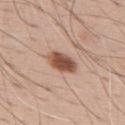Captured during whole-body skin photography for melanoma surveillance; the lesion was not biopsied.
Cropped from a total-body skin-imaging series; the visible field is about 15 mm.
The lesion's longest dimension is about 4 mm.
Located on the upper back.
Captured under white-light illumination.
A male patient about 70 years old.